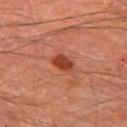Imaged during a routine full-body skin examination; the lesion was not biopsied and no histopathology is available.
The lesion is on the left thigh.
Longest diameter approximately 3 mm.
Imaged with cross-polarized lighting.
A close-up tile cropped from a whole-body skin photograph, about 15 mm across.
The patient is a male aged 63–67.
The lesion-visualizer software estimated a border-irregularity index near 2.5/10 and a within-lesion color-variation index near 2/10. And it measured lesion-presence confidence of about 100/100.